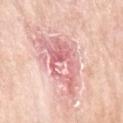location: the left upper arm | automated metrics: an area of roughly 20 mm² and an outline eccentricity of about 0.85 (0 = round, 1 = elongated); an automated nevus-likeness rating near 0 out of 100 and a detector confidence of about 65 out of 100 that the crop contains a lesion | patient: female, aged around 70 | image source: 15 mm crop, total-body photography | tile lighting: white-light | lesion size: about 7.5 mm.